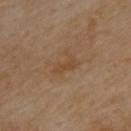Q: Was a biopsy performed?
A: total-body-photography surveillance lesion; no biopsy
Q: What are the patient's age and sex?
A: male, roughly 55 years of age
Q: Where on the body is the lesion?
A: the upper back
Q: What is the imaging modality?
A: ~15 mm crop, total-body skin-cancer survey
Q: Illumination type?
A: cross-polarized illumination
Q: Lesion size?
A: about 3 mm